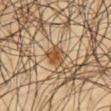The lesion was tiled from a total-body skin photograph and was not biopsied. A male patient, about 45 years old. Measured at roughly 3 mm in maximum diameter. The lesion is located on the chest. This is a cross-polarized tile. Cropped from a total-body skin-imaging series; the visible field is about 15 mm.